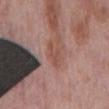<tbp_lesion>
  <biopsy_status>not biopsied; imaged during a skin examination</biopsy_status>
  <image>
    <source>total-body photography crop</source>
    <field_of_view_mm>15</field_of_view_mm>
  </image>
  <automated_metrics>
    <area_mm2_approx>4.5</area_mm2_approx>
    <shape_asymmetry>0.4</shape_asymmetry>
    <color_variation_0_10>1.5</color_variation_0_10>
    <peripheral_color_asymmetry>0.5</peripheral_color_asymmetry>
  </automated_metrics>
  <site>back</site>
  <lighting>white-light</lighting>
  <patient>
    <sex>male</sex>
    <age_approx>75</age_approx>
  </patient>
  <lesion_size>
    <long_diameter_mm_approx>3.5</long_diameter_mm_approx>
  </lesion_size>
</tbp_lesion>